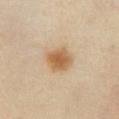Case summary:
* workup · no biopsy performed (imaged during a skin exam)
* image source · ~15 mm crop, total-body skin-cancer survey
* site · the chest
* image-analysis metrics · a footprint of about 8.5 mm², an eccentricity of roughly 0.45, and a symmetry-axis asymmetry near 0.2; internal color variation of about 3.5 on a 0–10 scale
* diameter · ~3.5 mm (longest diameter)
* patient · female, aged approximately 35
* tile lighting · cross-polarized illumination